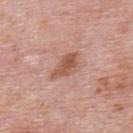follow-up=catalogued during a skin exam; not biopsied
diameter=about 4.5 mm
location=the upper back
imaging modality=total-body-photography crop, ~15 mm field of view
patient=male, aged approximately 75